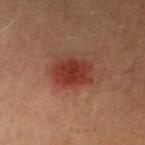Recorded during total-body skin imaging; not selected for excision or biopsy. Located on the left lower leg. A region of skin cropped from a whole-body photographic capture, roughly 15 mm wide. The patient is a male aged approximately 40.